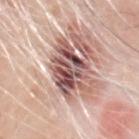  biopsy_status: not biopsied; imaged during a skin examination
  site: head or neck
  lesion_size:
    long_diameter_mm_approx: 5.5
  lighting: white-light
  automated_metrics:
    area_mm2_approx: 16.0
    eccentricity: 0.65
    shape_asymmetry: 0.35
    cielab_L: 53
    cielab_a: 22
    cielab_b: 21
    vs_skin_darker_L: 18.0
    vs_skin_contrast_norm: 11.5
    nevus_likeness_0_100: 20
  patient:
    sex: male
    age_approx: 80
  image:
    source: total-body photography crop
    field_of_view_mm: 15